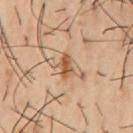Impression: Part of a total-body skin-imaging series; this lesion was reviewed on a skin check and was not flagged for biopsy. Clinical summary: Measured at roughly 3 mm in maximum diameter. The patient is a male about 55 years old. Automated image analysis of the tile measured a lesion color around L≈53 a*≈20 b*≈35 in CIELAB, a lesion–skin lightness drop of about 12, and a normalized border contrast of about 9. And it measured a within-lesion color-variation index near 5/10 and peripheral color asymmetry of about 2. The software also gave a nevus-likeness score of about 20/100 and a detector confidence of about 100 out of 100 that the crop contains a lesion. A lesion tile, about 15 mm wide, cut from a 3D total-body photograph. From the chest.